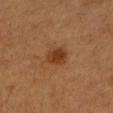Notes:
- workup — no biopsy performed (imaged during a skin exam)
- image source — 15 mm crop, total-body photography
- subject — female, roughly 55 years of age
- anatomic site — the right forearm
- tile lighting — cross-polarized illumination
- size — about 3 mm
- TBP lesion metrics — an average lesion color of about L≈30 a*≈20 b*≈31 (CIELAB) and a lesion–skin lightness drop of about 8; a border-irregularity index near 1.5/10; a lesion-detection confidence of about 100/100